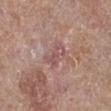{
  "lesion_size": {
    "long_diameter_mm_approx": 3.0
  },
  "site": "left leg",
  "image": {
    "source": "total-body photography crop",
    "field_of_view_mm": 15
  },
  "patient": {
    "sex": "male",
    "age_approx": 80
  }
}